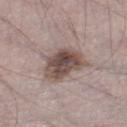Assessment: The lesion was photographed on a routine skin check and not biopsied; there is no pathology result. Context: The lesion is located on the right lower leg. A roughly 15 mm field-of-view crop from a total-body skin photograph. The subject is a male aged approximately 70. Captured under white-light illumination.